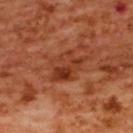Q: Was a biopsy performed?
A: catalogued during a skin exam; not biopsied
Q: Who is the patient?
A: female, in their mid- to late 50s
Q: What is the imaging modality?
A: 15 mm crop, total-body photography
Q: What is the anatomic site?
A: the back